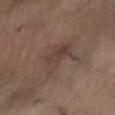<lesion>
<biopsy_status>not biopsied; imaged during a skin examination</biopsy_status>
<site>left lower leg</site>
<lesion_size>
  <long_diameter_mm_approx>5.0</long_diameter_mm_approx>
</lesion_size>
<automated_metrics>
  <area_mm2_approx>11.0</area_mm2_approx>
  <shape_asymmetry>0.45</shape_asymmetry>
  <border_irregularity_0_10>6.0</border_irregularity_0_10>
  <color_variation_0_10>4.0</color_variation_0_10>
  <peripheral_color_asymmetry>1.5</peripheral_color_asymmetry>
  <nevus_likeness_0_100>0</nevus_likeness_0_100>
</automated_metrics>
<image>
  <source>total-body photography crop</source>
  <field_of_view_mm>15</field_of_view_mm>
</image>
</lesion>